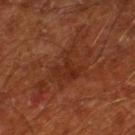This lesion was catalogued during total-body skin photography and was not selected for biopsy.
From the right lower leg.
A close-up tile cropped from a whole-body skin photograph, about 15 mm across.
The lesion's longest dimension is about 6 mm.
A male subject, aged around 65.